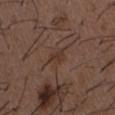The lesion was tiled from a total-body skin photograph and was not biopsied. The recorded lesion diameter is about 2.5 mm. Automated tile analysis of the lesion measured an area of roughly 3.5 mm², an outline eccentricity of about 0.75 (0 = round, 1 = elongated), and two-axis asymmetry of about 0.4. A region of skin cropped from a whole-body photographic capture, roughly 15 mm wide. From the chest. The patient is a male aged 48 to 52.